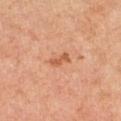{
  "biopsy_status": "not biopsied; imaged during a skin examination",
  "image": {
    "source": "total-body photography crop",
    "field_of_view_mm": 15
  },
  "lesion_size": {
    "long_diameter_mm_approx": 2.5
  },
  "site": "left thigh",
  "lighting": "cross-polarized",
  "patient": {
    "sex": "female"
  }
}